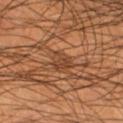  biopsy_status: not biopsied; imaged during a skin examination
  site: leg
  lesion_size:
    long_diameter_mm_approx: 2.5
  lighting: cross-polarized
  patient:
    sex: male
    age_approx: 55
  automated_metrics:
    cielab_L: 39
    cielab_a: 21
    cielab_b: 31
    vs_skin_darker_L: 7.0
    vs_skin_contrast_norm: 6.0
    border_irregularity_0_10: 3.0
    color_variation_0_10: 2.5
    peripheral_color_asymmetry: 1.0
  image:
    source: total-body photography crop
    field_of_view_mm: 15